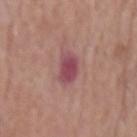biopsy_status: not biopsied; imaged during a skin examination
patient:
  sex: male
  age_approx: 70
image:
  source: total-body photography crop
  field_of_view_mm: 15
site: head or neck
lighting: white-light
lesion_size:
  long_diameter_mm_approx: 3.0